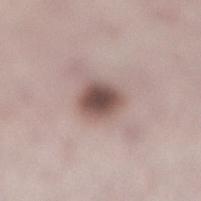No biopsy was performed on this lesion — it was imaged during a full skin examination and was not determined to be concerning. A 15 mm close-up extracted from a 3D total-body photography capture. The recorded lesion diameter is about 3.5 mm. Imaged with white-light lighting. From the right lower leg. A female subject, roughly 50 years of age.